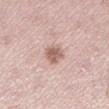  biopsy_status: not biopsied; imaged during a skin examination
  patient:
    sex: female
    age_approx: 50
  site: left lower leg
  image:
    source: total-body photography crop
    field_of_view_mm: 15
  lighting: white-light